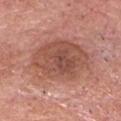Q: Was a biopsy performed?
A: no biopsy performed (imaged during a skin exam)
Q: What is the anatomic site?
A: the head or neck
Q: What did automated image analysis measure?
A: a footprint of about 31 mm² and a symmetry-axis asymmetry near 0.15; an average lesion color of about L≈51 a*≈24 b*≈28 (CIELAB), a lesion–skin lightness drop of about 10, and a lesion-to-skin contrast of about 7.5 (normalized; higher = more distinct); border irregularity of about 2 on a 0–10 scale, a within-lesion color-variation index near 4.5/10, and peripheral color asymmetry of about 1.5; an automated nevus-likeness rating near 5 out of 100 and lesion-presence confidence of about 100/100
Q: Illumination type?
A: white-light illumination
Q: What is the imaging modality?
A: total-body-photography crop, ~15 mm field of view
Q: What are the patient's age and sex?
A: male, aged approximately 80